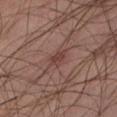The lesion was photographed on a routine skin check and not biopsied; there is no pathology result. A 15 mm close-up tile from a total-body photography series done for melanoma screening. Imaged with cross-polarized lighting. About 2.5 mm across. Located on the right thigh. A male patient aged approximately 45. Automated tile analysis of the lesion measured a footprint of about 4 mm² and a shape-asymmetry score of about 0.2 (0 = symmetric). The software also gave a mean CIELAB color near L≈38 a*≈19 b*≈21 and about 7 CIELAB-L* units darker than the surrounding skin.